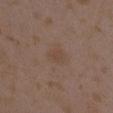Q: Was this lesion biopsied?
A: no biopsy performed (imaged during a skin exam)
Q: How large is the lesion?
A: about 2.5 mm
Q: What lighting was used for the tile?
A: white-light illumination
Q: What did automated image analysis measure?
A: a footprint of about 4 mm² and two-axis asymmetry of about 0.25; a classifier nevus-likeness of about 0/100 and a detector confidence of about 100 out of 100 that the crop contains a lesion
Q: Lesion location?
A: the right upper arm
Q: What are the patient's age and sex?
A: female, aged around 35
Q: What kind of image is this?
A: ~15 mm crop, total-body skin-cancer survey